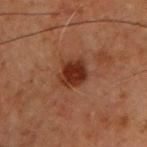Impression:
No biopsy was performed on this lesion — it was imaged during a full skin examination and was not determined to be concerning.
Clinical summary:
A male patient aged around 60. On the chest. Measured at roughly 3 mm in maximum diameter. Captured under cross-polarized illumination. A roughly 15 mm field-of-view crop from a total-body skin photograph.